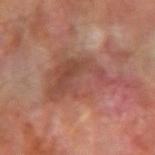No biopsy was performed on this lesion — it was imaged during a full skin examination and was not determined to be concerning.
An algorithmic analysis of the crop reported a lesion area of about 23 mm², a shape eccentricity near 0.75, and a symmetry-axis asymmetry near 0.55. And it measured border irregularity of about 9 on a 0–10 scale and internal color variation of about 4.5 on a 0–10 scale. It also reported a nevus-likeness score of about 0/100 and a lesion-detection confidence of about 75/100.
A male patient approximately 70 years of age.
A 15 mm close-up extracted from a 3D total-body photography capture.
Longest diameter approximately 8.5 mm.
Imaged with cross-polarized lighting.
Located on the left forearm.